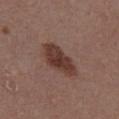Assessment: Captured during whole-body skin photography for melanoma surveillance; the lesion was not biopsied. Image and clinical context: On the chest. A lesion tile, about 15 mm wide, cut from a 3D total-body photograph. A male subject, in their 40s. The tile uses white-light illumination. Measured at roughly 5.5 mm in maximum diameter.